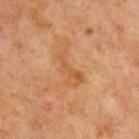{"biopsy_status": "not biopsied; imaged during a skin examination", "patient": {"sex": "male", "age_approx": 65}, "site": "chest", "image": {"source": "total-body photography crop", "field_of_view_mm": 15}, "lesion_size": {"long_diameter_mm_approx": 4.5}, "lighting": "cross-polarized"}